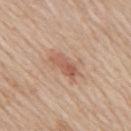Image and clinical context:
A male patient, aged approximately 60. Automated image analysis of the tile measured a lesion area of about 6.5 mm², an outline eccentricity of about 0.9 (0 = round, 1 = elongated), and two-axis asymmetry of about 0.2. From the mid back. A lesion tile, about 15 mm wide, cut from a 3D total-body photograph. This is a white-light tile.